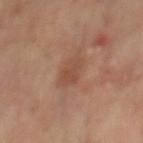Recorded during total-body skin imaging; not selected for excision or biopsy.
Imaged with cross-polarized lighting.
On the mid back.
Longest diameter approximately 3.5 mm.
A 15 mm close-up extracted from a 3D total-body photography capture.
The subject is a male aged around 65.